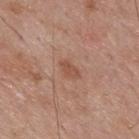Clinical impression: Recorded during total-body skin imaging; not selected for excision or biopsy. Background: The patient is a male roughly 70 years of age. The lesion's longest dimension is about 3 mm. Captured under white-light illumination. From the upper back. Automated image analysis of the tile measured an area of roughly 3.5 mm², an outline eccentricity of about 0.85 (0 = round, 1 = elongated), and a shape-asymmetry score of about 0.35 (0 = symmetric). The analysis additionally found a lesion color around L≈51 a*≈22 b*≈30 in CIELAB and a normalized lesion–skin contrast near 6. The software also gave a classifier nevus-likeness of about 40/100 and lesion-presence confidence of about 100/100. A roughly 15 mm field-of-view crop from a total-body skin photograph.